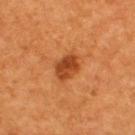No biopsy was performed on this lesion — it was imaged during a full skin examination and was not determined to be concerning.
A region of skin cropped from a whole-body photographic capture, roughly 15 mm wide.
The lesion is located on the upper back.
About 3 mm across.
The tile uses cross-polarized illumination.
A male patient, approximately 55 years of age.